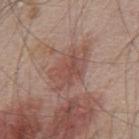Notes:
• notes: no biopsy performed (imaged during a skin exam)
• TBP lesion metrics: a border-irregularity index near 4/10, a color-variation rating of about 4/10, and a peripheral color-asymmetry measure near 1; a nevus-likeness score of about 0/100 and a detector confidence of about 95 out of 100 that the crop contains a lesion
• image: total-body-photography crop, ~15 mm field of view
• diameter: about 6 mm
• body site: the mid back
• tile lighting: white-light
• patient: male, aged 48–52